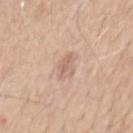Clinical impression:
Part of a total-body skin-imaging series; this lesion was reviewed on a skin check and was not flagged for biopsy.
Clinical summary:
The lesion is located on the mid back. A male patient, aged approximately 65. About 3.5 mm across. An algorithmic analysis of the crop reported a footprint of about 5 mm², a shape eccentricity near 0.9, and a symmetry-axis asymmetry near 0.25. And it measured an automated nevus-likeness rating near 0 out of 100 and lesion-presence confidence of about 100/100. This is a white-light tile. A region of skin cropped from a whole-body photographic capture, roughly 15 mm wide.